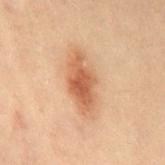| feature | finding |
|---|---|
| workup | imaged on a skin check; not biopsied |
| diameter | ≈6.5 mm |
| automated lesion analysis | a classifier nevus-likeness of about 90/100 |
| acquisition | total-body-photography crop, ~15 mm field of view |
| anatomic site | the chest |
| patient | female, aged 18 to 22 |
| lighting | cross-polarized illumination |